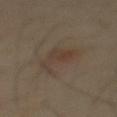Imaged during a routine full-body skin examination; the lesion was not biopsied and no histopathology is available.